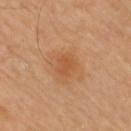Q: Was a biopsy performed?
A: total-body-photography surveillance lesion; no biopsy
Q: What kind of image is this?
A: total-body-photography crop, ~15 mm field of view
Q: Lesion location?
A: the arm
Q: Lesion size?
A: ~2.5 mm (longest diameter)
Q: How was the tile lit?
A: cross-polarized illumination